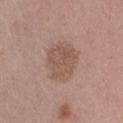workup: no biopsy performed (imaged during a skin exam)
image-analysis metrics: a mean CIELAB color near L≈54 a*≈18 b*≈25 and a normalized lesion–skin contrast near 6; a border-irregularity rating of about 2/10, internal color variation of about 2.5 on a 0–10 scale, and radial color variation of about 1
lighting: white-light
patient: female, aged 43 to 47
imaging modality: 15 mm crop, total-body photography
body site: the right thigh